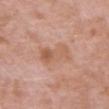Findings:
* notes: catalogued during a skin exam; not biopsied
* body site: the chest
* acquisition: total-body-photography crop, ~15 mm field of view
* diameter: about 4 mm
* patient: female, aged 68–72
* lighting: white-light illumination
* automated lesion analysis: a classifier nevus-likeness of about 0/100